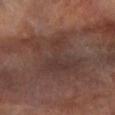No biopsy was performed on this lesion — it was imaged during a full skin examination and was not determined to be concerning.
A close-up tile cropped from a whole-body skin photograph, about 15 mm across.
The recorded lesion diameter is about 8.5 mm.
A female subject aged approximately 65.
Located on the left arm.
The tile uses cross-polarized illumination.